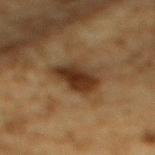patient: male, aged 83 or over
acquisition: total-body-photography crop, ~15 mm field of view
body site: the back
size: ≈5 mm
lighting: cross-polarized
image-analysis metrics: a lesion area of about 11 mm² and an eccentricity of roughly 0.8; an average lesion color of about L≈29 a*≈16 b*≈27 (CIELAB) and a normalized lesion–skin contrast near 11; a color-variation rating of about 4/10 and a peripheral color-asymmetry measure near 1; a classifier nevus-likeness of about 95/100